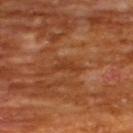Q: How large is the lesion?
A: ≈2.5 mm
Q: Who is the patient?
A: male, about 65 years old
Q: How was the tile lit?
A: cross-polarized illumination
Q: Lesion location?
A: the upper back
Q: What kind of image is this?
A: 15 mm crop, total-body photography